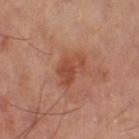Captured during whole-body skin photography for melanoma surveillance; the lesion was not biopsied. The tile uses cross-polarized illumination. From the right thigh. An algorithmic analysis of the crop reported a lesion area of about 6.5 mm², an eccentricity of roughly 0.8, and a shape-asymmetry score of about 0.45 (0 = symmetric). And it measured an average lesion color of about L≈48 a*≈27 b*≈32 (CIELAB), about 8 CIELAB-L* units darker than the surrounding skin, and a lesion-to-skin contrast of about 6.5 (normalized; higher = more distinct). The software also gave internal color variation of about 3 on a 0–10 scale and a peripheral color-asymmetry measure near 1. The lesion's longest dimension is about 4 mm. A region of skin cropped from a whole-body photographic capture, roughly 15 mm wide.